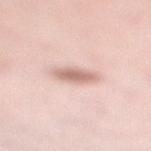notes = total-body-photography surveillance lesion; no biopsy
lesion diameter = ≈3.5 mm
automated metrics = a mean CIELAB color near L≈68 a*≈20 b*≈25 and a normalized lesion–skin contrast near 7.5; a nevus-likeness score of about 95/100 and a detector confidence of about 100 out of 100 that the crop contains a lesion
body site = the leg
image = ~15 mm tile from a whole-body skin photo
patient = female, aged 28 to 32